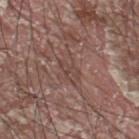Imaged during a routine full-body skin examination; the lesion was not biopsied and no histopathology is available.
This image is a 15 mm lesion crop taken from a total-body photograph.
The tile uses white-light illumination.
A male patient aged approximately 40.
From the upper back.